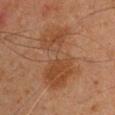Case summary:
* follow-up · imaged on a skin check; not biopsied
* illumination · cross-polarized illumination
* diameter · ≈8 mm
* patient · male, in their mid-40s
* anatomic site · the front of the torso
* imaging modality · ~15 mm crop, total-body skin-cancer survey